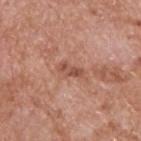workup: total-body-photography surveillance lesion; no biopsy | automated metrics: an outline eccentricity of about 0.9 (0 = round, 1 = elongated) | subject: male, aged around 60 | image source: 15 mm crop, total-body photography | anatomic site: the upper back | illumination: white-light illumination | lesion size: ≈3 mm.